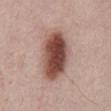The lesion was photographed on a routine skin check and not biopsied; there is no pathology result.